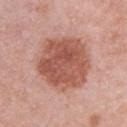* follow-up · catalogued during a skin exam; not biopsied
* subject · female, about 65 years old
* TBP lesion metrics · an average lesion color of about L≈54 a*≈25 b*≈28 (CIELAB), about 13 CIELAB-L* units darker than the surrounding skin, and a lesion-to-skin contrast of about 9 (normalized; higher = more distinct); a border-irregularity rating of about 1.5/10, a color-variation rating of about 5/10, and radial color variation of about 2
* body site · the right upper arm
* lesion size · ~6.5 mm (longest diameter)
* image source · ~15 mm crop, total-body skin-cancer survey
* illumination · white-light